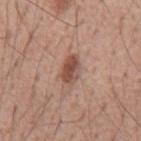biopsy status: no biopsy performed (imaged during a skin exam)
acquisition: ~15 mm tile from a whole-body skin photo
TBP lesion metrics: a lesion area of about 5.5 mm², an outline eccentricity of about 0.8 (0 = round, 1 = elongated), and a shape-asymmetry score of about 0.15 (0 = symmetric); an average lesion color of about L≈50 a*≈21 b*≈27 (CIELAB), about 12 CIELAB-L* units darker than the surrounding skin, and a normalized border contrast of about 8.5
illumination: white-light
site: the mid back
subject: male, roughly 55 years of age